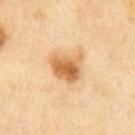Background:
About 4 mm across. A male subject aged 68–72. The tile uses cross-polarized illumination. The total-body-photography lesion software estimated a lesion color around L≈52 a*≈18 b*≈36 in CIELAB, about 11 CIELAB-L* units darker than the surrounding skin, and a normalized border contrast of about 9. And it measured a border-irregularity rating of about 3/10 and a color-variation rating of about 5/10. It also reported a classifier nevus-likeness of about 95/100. Located on the chest. A roughly 15 mm field-of-view crop from a total-body skin photograph.